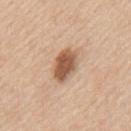Impression: The lesion was photographed on a routine skin check and not biopsied; there is no pathology result. Image and clinical context: A male patient approximately 60 years of age. A 15 mm crop from a total-body photograph taken for skin-cancer surveillance. From the mid back.